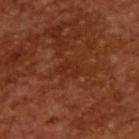Captured during whole-body skin photography for melanoma surveillance; the lesion was not biopsied. The recorded lesion diameter is about 2.5 mm. A male patient in their mid- to late 60s. The lesion-visualizer software estimated an eccentricity of roughly 0.7 and two-axis asymmetry of about 0.25. The analysis additionally found a border-irregularity rating of about 3/10, internal color variation of about 1.5 on a 0–10 scale, and peripheral color asymmetry of about 0.5. The analysis additionally found a classifier nevus-likeness of about 0/100 and a detector confidence of about 95 out of 100 that the crop contains a lesion. A close-up tile cropped from a whole-body skin photograph, about 15 mm across. This is a cross-polarized tile.